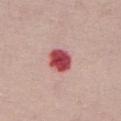biopsy_status: not biopsied; imaged during a skin examination
lighting: white-light
image:
  source: total-body photography crop
  field_of_view_mm: 15
patient:
  sex: female
  age_approx: 65
site: chest
automated_metrics:
  cielab_L: 49
  cielab_a: 37
  cielab_b: 24
  vs_skin_darker_L: 20.0
  vs_skin_contrast_norm: 13.0
  border_irregularity_0_10: 1.0
  color_variation_0_10: 4.0
  peripheral_color_asymmetry: 1.0